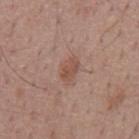No biopsy was performed on this lesion — it was imaged during a full skin examination and was not determined to be concerning. From the back. Captured under white-light illumination. Measured at roughly 3 mm in maximum diameter. A 15 mm close-up extracted from a 3D total-body photography capture. A male subject aged approximately 60. The lesion-visualizer software estimated a lesion area of about 4.5 mm², a shape eccentricity near 0.8, and a symmetry-axis asymmetry near 0.2. And it measured border irregularity of about 2 on a 0–10 scale, a color-variation rating of about 2/10, and peripheral color asymmetry of about 0.5. The analysis additionally found a nevus-likeness score of about 55/100 and a lesion-detection confidence of about 100/100.